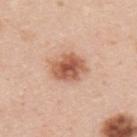Q: Was a biopsy performed?
A: total-body-photography surveillance lesion; no biopsy
Q: Where on the body is the lesion?
A: the upper back
Q: What is the lesion's diameter?
A: about 4.5 mm
Q: What is the imaging modality?
A: total-body-photography crop, ~15 mm field of view
Q: What are the patient's age and sex?
A: male, about 45 years old